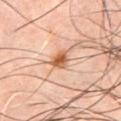Clinical impression:
Recorded during total-body skin imaging; not selected for excision or biopsy.
Background:
Imaged with cross-polarized lighting. Cropped from a total-body skin-imaging series; the visible field is about 15 mm. A male subject, aged 43 to 47. Located on the chest. The total-body-photography lesion software estimated a lesion area of about 4 mm², a shape eccentricity near 0.7, and a shape-asymmetry score of about 0.3 (0 = symmetric). The analysis additionally found an automated nevus-likeness rating near 95 out of 100 and lesion-presence confidence of about 100/100.